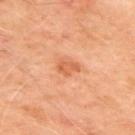The subject is a male aged around 65.
On the upper back.
Cropped from a whole-body photographic skin survey; the tile spans about 15 mm.
This is a cross-polarized tile.
The lesion's longest dimension is about 2.5 mm.
Automated image analysis of the tile measured a lesion color around L≈59 a*≈28 b*≈39 in CIELAB and a normalized border contrast of about 6.5.